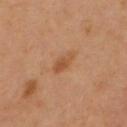Impression:
This lesion was catalogued during total-body skin photography and was not selected for biopsy.
Context:
Captured under cross-polarized illumination. The lesion is on the front of the torso. A lesion tile, about 15 mm wide, cut from a 3D total-body photograph. About 3 mm across. Automated tile analysis of the lesion measured an area of roughly 3.5 mm². It also reported a normalized lesion–skin contrast near 6.5. It also reported a border-irregularity index near 2.5/10 and peripheral color asymmetry of about 0.5. The patient is a male aged 53–57.